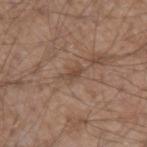Case summary:
• biopsy status: no biopsy performed (imaged during a skin exam)
• imaging modality: ~15 mm crop, total-body skin-cancer survey
• tile lighting: white-light
• location: the left forearm
• patient: male, in their mid- to late 50s